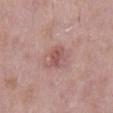The lesion's longest dimension is about 3.5 mm.
On the mid back.
Captured under white-light illumination.
The subject is a male aged approximately 55.
A region of skin cropped from a whole-body photographic capture, roughly 15 mm wide.